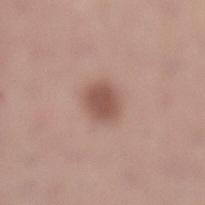Case summary:
– follow-up · no biopsy performed (imaged during a skin exam)
– illumination · white-light illumination
– body site · the leg
– imaging modality · ~15 mm tile from a whole-body skin photo
– lesion diameter · about 3 mm
– patient · female, roughly 30 years of age
– automated lesion analysis · an average lesion color of about L≈52 a*≈21 b*≈24 (CIELAB), about 11 CIELAB-L* units darker than the surrounding skin, and a normalized lesion–skin contrast near 8; an automated nevus-likeness rating near 95 out of 100 and a lesion-detection confidence of about 100/100